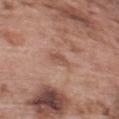patient = male, aged 68 to 72 | location = the upper back | acquisition = 15 mm crop, total-body photography | lesion size = ≈2.5 mm.